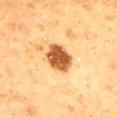biopsy_status: not biopsied; imaged during a skin examination
site: mid back
image:
  source: total-body photography crop
  field_of_view_mm: 15
patient:
  sex: male
  age_approx: 60
automated_metrics:
  cielab_L: 60
  cielab_a: 26
  cielab_b: 45
  vs_skin_darker_L: 21.0
  vs_skin_contrast_norm: 12.0
  color_variation_0_10: 4.5
  peripheral_color_asymmetry: 1.5
  nevus_likeness_0_100: 90
  lesion_detection_confidence_0_100: 100